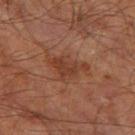<record>
  <biopsy_status>not biopsied; imaged during a skin examination</biopsy_status>
  <patient>
    <sex>male</sex>
    <age_approx>60</age_approx>
  </patient>
  <lighting>cross-polarized</lighting>
  <image>
    <source>total-body photography crop</source>
    <field_of_view_mm>15</field_of_view_mm>
  </image>
  <site>right leg</site>
</record>